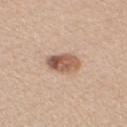Captured during whole-body skin photography for melanoma surveillance; the lesion was not biopsied. Cropped from a total-body skin-imaging series; the visible field is about 15 mm. The lesion is located on the chest. A female patient, aged around 60.